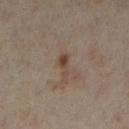| field | value |
|---|---|
| biopsy status | no biopsy performed (imaged during a skin exam) |
| image | 15 mm crop, total-body photography |
| anatomic site | the left lower leg |
| subject | female, roughly 35 years of age |
| automated lesion analysis | a border-irregularity index near 5/10, internal color variation of about 1 on a 0–10 scale, and radial color variation of about 0; a lesion-detection confidence of about 100/100 |
| lesion size | ≈3 mm |